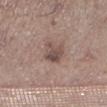Q: Is there a histopathology result?
A: catalogued during a skin exam; not biopsied
Q: What did automated image analysis measure?
A: a footprint of about 6 mm², an eccentricity of roughly 0.65, and two-axis asymmetry of about 0.2; a lesion color around L≈49 a*≈16 b*≈21 in CIELAB, about 10 CIELAB-L* units darker than the surrounding skin, and a lesion-to-skin contrast of about 7.5 (normalized; higher = more distinct); border irregularity of about 2.5 on a 0–10 scale and peripheral color asymmetry of about 1.5; a classifier nevus-likeness of about 10/100 and a detector confidence of about 100 out of 100 that the crop contains a lesion
Q: What is the anatomic site?
A: the left lower leg
Q: What kind of image is this?
A: total-body-photography crop, ~15 mm field of view
Q: What are the patient's age and sex?
A: male, about 65 years old